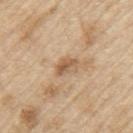Clinical impression: The lesion was photographed on a routine skin check and not biopsied; there is no pathology result. Context: The lesion is located on the left upper arm. A close-up tile cropped from a whole-body skin photograph, about 15 mm across. A male subject, in their 70s. The recorded lesion diameter is about 2.5 mm.